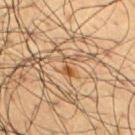| feature | finding |
|---|---|
| illumination | cross-polarized |
| patient | male, aged around 60 |
| location | the right upper arm |
| acquisition | total-body-photography crop, ~15 mm field of view |
| image-analysis metrics | border irregularity of about 5 on a 0–10 scale, a within-lesion color-variation index near 0.5/10, and peripheral color asymmetry of about 0; a nevus-likeness score of about 5/100 |
| lesion size | ~2.5 mm (longest diameter) |